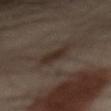{
  "biopsy_status": "not biopsied; imaged during a skin examination",
  "lighting": "cross-polarized",
  "site": "mid back",
  "lesion_size": {
    "long_diameter_mm_approx": 4.0
  },
  "patient": {
    "sex": "male",
    "age_approx": 50
  },
  "image": {
    "source": "total-body photography crop",
    "field_of_view_mm": 15
  }
}